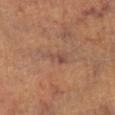Findings:
– workup: catalogued during a skin exam; not biopsied
– body site: the right lower leg
– image: ~15 mm tile from a whole-body skin photo
– tile lighting: cross-polarized illumination
– patient: female, roughly 65 years of age
– automated lesion analysis: an outline eccentricity of about 0.9 (0 = round, 1 = elongated) and a symmetry-axis asymmetry near 0.4; border irregularity of about 4.5 on a 0–10 scale, a within-lesion color-variation index near 3/10, and peripheral color asymmetry of about 1; a nevus-likeness score of about 0/100 and a lesion-detection confidence of about 90/100